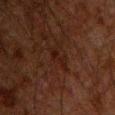Longest diameter approximately 3 mm.
An algorithmic analysis of the crop reported a classifier nevus-likeness of about 0/100.
The subject is a male aged around 60.
A roughly 15 mm field-of-view crop from a total-body skin photograph.
Imaged with cross-polarized lighting.
From the chest.